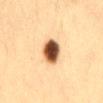Impression: Imaged during a routine full-body skin examination; the lesion was not biopsied and no histopathology is available. Background: The tile uses cross-polarized illumination. On the mid back. A female patient roughly 30 years of age. Cropped from a total-body skin-imaging series; the visible field is about 15 mm. Automated tile analysis of the lesion measured a lesion area of about 9 mm², an outline eccentricity of about 0.8 (0 = round, 1 = elongated), and two-axis asymmetry of about 0.15. The analysis additionally found a border-irregularity index near 1.5/10 and a color-variation rating of about 10/10.